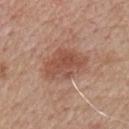Clinical impression:
No biopsy was performed on this lesion — it was imaged during a full skin examination and was not determined to be concerning.
Image and clinical context:
The tile uses white-light illumination. A region of skin cropped from a whole-body photographic capture, roughly 15 mm wide. The recorded lesion diameter is about 5 mm. A male subject, aged 73 to 77. The lesion is on the chest.